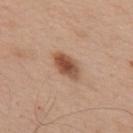Q: Was this lesion biopsied?
A: imaged on a skin check; not biopsied
Q: How was this image acquired?
A: ~15 mm crop, total-body skin-cancer survey
Q: What are the patient's age and sex?
A: male, aged 53–57
Q: What lighting was used for the tile?
A: white-light
Q: Lesion size?
A: about 4 mm
Q: What is the anatomic site?
A: the upper back